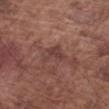Findings:
• image — ~15 mm tile from a whole-body skin photo
• location — the right forearm
• lesion diameter — ~3 mm (longest diameter)
• lighting — white-light illumination
• subject — male, in their mid-70s